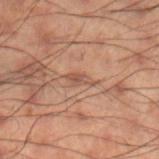The lesion was photographed on a routine skin check and not biopsied; there is no pathology result. On the right lower leg. A male patient roughly 50 years of age. A roughly 15 mm field-of-view crop from a total-body skin photograph. The recorded lesion diameter is about 3 mm. Imaged with cross-polarized lighting.